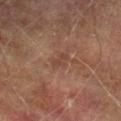- biopsy status: total-body-photography surveillance lesion; no biopsy
- image-analysis metrics: a lesion color around L≈34 a*≈17 b*≈23 in CIELAB, a lesion–skin lightness drop of about 5, and a normalized border contrast of about 5; a nevus-likeness score of about 0/100 and lesion-presence confidence of about 100/100
- diameter: ~2.5 mm (longest diameter)
- lighting: cross-polarized illumination
- subject: male, roughly 75 years of age
- imaging modality: total-body-photography crop, ~15 mm field of view
- body site: the right lower leg